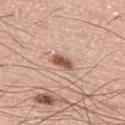Captured during whole-body skin photography for melanoma surveillance; the lesion was not biopsied. A male subject, roughly 75 years of age. A 15 mm crop from a total-body photograph taken for skin-cancer surveillance. On the upper back.